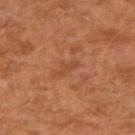biopsy status: total-body-photography surveillance lesion; no biopsy
subject: male, aged around 55
TBP lesion metrics: a footprint of about 2.5 mm² and a shape-asymmetry score of about 0.4 (0 = symmetric); a border-irregularity index near 4.5/10, a within-lesion color-variation index near 0/10, and radial color variation of about 0; a lesion-detection confidence of about 100/100
acquisition: ~15 mm tile from a whole-body skin photo
lighting: cross-polarized
anatomic site: the right upper arm